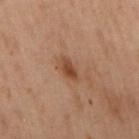The lesion was photographed on a routine skin check and not biopsied; there is no pathology result. Captured under cross-polarized illumination. Approximately 2.5 mm at its widest. The subject is a female roughly 55 years of age. The lesion is located on the left arm. Automated image analysis of the tile measured border irregularity of about 2.5 on a 0–10 scale, a color-variation rating of about 2.5/10, and a peripheral color-asymmetry measure near 1. A 15 mm close-up tile from a total-body photography series done for melanoma screening.